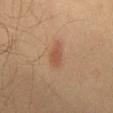On the chest.
The patient is a male aged 53 to 57.
A roughly 15 mm field-of-view crop from a total-body skin photograph.